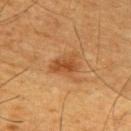Notes:
• notes — no biopsy performed (imaged during a skin exam)
• image — ~15 mm tile from a whole-body skin photo
• subject — male, aged 58–62
• site — the left upper arm